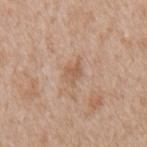– lesion size · ≈3 mm
– lighting · white-light
– anatomic site · the back
– subject · male, about 65 years old
– acquisition · total-body-photography crop, ~15 mm field of view